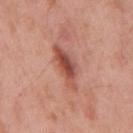The lesion was photographed on a routine skin check and not biopsied; there is no pathology result. Captured under white-light illumination. About 5.5 mm across. A 15 mm close-up extracted from a 3D total-body photography capture. A male subject approximately 55 years of age. The lesion is located on the mid back.